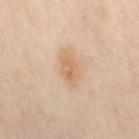notes = no biopsy performed (imaged during a skin exam)
site = the lower back
acquisition = ~15 mm crop, total-body skin-cancer survey
illumination = cross-polarized illumination
diameter = ~3 mm (longest diameter)
TBP lesion metrics = border irregularity of about 2.5 on a 0–10 scale, internal color variation of about 3 on a 0–10 scale, and peripheral color asymmetry of about 1; a detector confidence of about 100 out of 100 that the crop contains a lesion
subject = female, aged 38–42